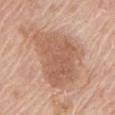Part of a total-body skin-imaging series; this lesion was reviewed on a skin check and was not flagged for biopsy. The subject is a male aged around 65. Measured at roughly 7.5 mm in maximum diameter. This is a white-light tile. Cropped from a whole-body photographic skin survey; the tile spans about 15 mm. On the chest.